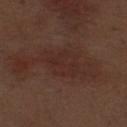Assessment:
Captured during whole-body skin photography for melanoma surveillance; the lesion was not biopsied.
Context:
From the left thigh. A male patient, about 70 years old. Longest diameter approximately 4.5 mm. This is a white-light tile. A close-up tile cropped from a whole-body skin photograph, about 15 mm across. The total-body-photography lesion software estimated a footprint of about 10 mm², a shape eccentricity near 0.7, and two-axis asymmetry of about 0.3. The analysis additionally found a lesion color around L≈28 a*≈19 b*≈22 in CIELAB, about 4 CIELAB-L* units darker than the surrounding skin, and a normalized lesion–skin contrast near 5.